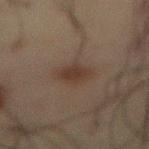Q: Is there a histopathology result?
A: imaged on a skin check; not biopsied
Q: What are the patient's age and sex?
A: male, about 60 years old
Q: What is the anatomic site?
A: the abdomen
Q: How was the tile lit?
A: cross-polarized illumination
Q: What did automated image analysis measure?
A: a lesion color around L≈27 a*≈13 b*≈19 in CIELAB, about 6 CIELAB-L* units darker than the surrounding skin, and a lesion-to-skin contrast of about 7 (normalized; higher = more distinct)
Q: What is the imaging modality?
A: ~15 mm tile from a whole-body skin photo
Q: How large is the lesion?
A: ~3 mm (longest diameter)